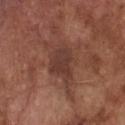Recorded during total-body skin imaging; not selected for excision or biopsy.
The total-body-photography lesion software estimated an area of roughly 16 mm² and an outline eccentricity of about 0.85 (0 = round, 1 = elongated). The analysis additionally found a lesion–skin lightness drop of about 8 and a normalized border contrast of about 7. The software also gave a classifier nevus-likeness of about 0/100.
About 7 mm across.
A male patient approximately 75 years of age.
The lesion is located on the chest.
This image is a 15 mm lesion crop taken from a total-body photograph.
Imaged with white-light lighting.